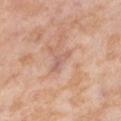This lesion was catalogued during total-body skin photography and was not selected for biopsy.